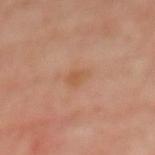Q: Is there a histopathology result?
A: total-body-photography surveillance lesion; no biopsy
Q: How was this image acquired?
A: ~15 mm tile from a whole-body skin photo
Q: What is the anatomic site?
A: the back
Q: Lesion size?
A: about 2.5 mm
Q: Patient demographics?
A: female, approximately 50 years of age
Q: What lighting was used for the tile?
A: cross-polarized